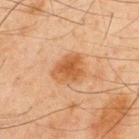{"biopsy_status": "not biopsied; imaged during a skin examination", "image": {"source": "total-body photography crop", "field_of_view_mm": 15}, "patient": {"sex": "male", "age_approx": 45}, "site": "back"}